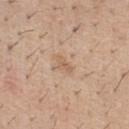Captured under white-light illumination. A 15 mm crop from a total-body photograph taken for skin-cancer surveillance. A male subject about 40 years old. Located on the chest.